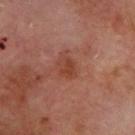Impression: Part of a total-body skin-imaging series; this lesion was reviewed on a skin check and was not flagged for biopsy. Image and clinical context: The lesion-visualizer software estimated a lesion color around L≈41 a*≈25 b*≈29 in CIELAB, a lesion–skin lightness drop of about 7, and a normalized border contrast of about 6.5. The software also gave border irregularity of about 2.5 on a 0–10 scale, a color-variation rating of about 1.5/10, and peripheral color asymmetry of about 0.5. A close-up tile cropped from a whole-body skin photograph, about 15 mm across. The tile uses cross-polarized illumination. The lesion is on the upper back. The recorded lesion diameter is about 2.5 mm. The patient is a male aged approximately 70.